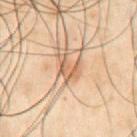| field | value |
|---|---|
| biopsy status | total-body-photography surveillance lesion; no biopsy |
| anatomic site | the abdomen |
| tile lighting | cross-polarized illumination |
| patient | male, about 50 years old |
| imaging modality | total-body-photography crop, ~15 mm field of view |
| lesion size | about 3 mm |
| image-analysis metrics | border irregularity of about 4.5 on a 0–10 scale, a within-lesion color-variation index near 3/10, and a peripheral color-asymmetry measure near 1 |